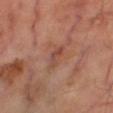Notes:
– follow-up: total-body-photography surveillance lesion; no biopsy